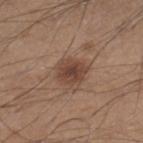Clinical summary: A 15 mm crop from a total-body photograph taken for skin-cancer surveillance. From the right lower leg. The patient is a male aged approximately 45.